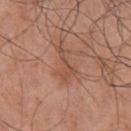- follow-up — total-body-photography surveillance lesion; no biopsy
- patient — male, aged 68 to 72
- automated metrics — a footprint of about 4 mm², a shape eccentricity near 0.95, and a symmetry-axis asymmetry near 0.7; a nevus-likeness score of about 5/100
- lighting — white-light
- location — the chest
- diameter — about 4 mm
- image source — total-body-photography crop, ~15 mm field of view